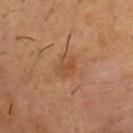From the chest.
Automated image analysis of the tile measured a nevus-likeness score of about 10/100 and a detector confidence of about 100 out of 100 that the crop contains a lesion.
A male subject, aged approximately 50.
A 15 mm close-up tile from a total-body photography series done for melanoma screening.